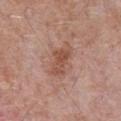Recorded during total-body skin imaging; not selected for excision or biopsy. Imaged with white-light lighting. Cropped from a whole-body photographic skin survey; the tile spans about 15 mm. A male patient, in their 70s. Approximately 4.5 mm at its widest. An algorithmic analysis of the crop reported a footprint of about 7.5 mm², an outline eccentricity of about 0.85 (0 = round, 1 = elongated), and two-axis asymmetry of about 0.35. The analysis additionally found a lesion color around L≈51 a*≈22 b*≈29 in CIELAB, a lesion–skin lightness drop of about 9, and a normalized border contrast of about 7. The lesion is located on the chest.